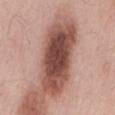workup: no biopsy performed (imaged during a skin exam)
lesion size: about 10 mm
location: the mid back
automated metrics: an outline eccentricity of about 0.95 (0 = round, 1 = elongated) and two-axis asymmetry of about 0.2; border irregularity of about 3.5 on a 0–10 scale, internal color variation of about 6.5 on a 0–10 scale, and a peripheral color-asymmetry measure near 2
patient: male, aged 53 to 57
image: ~15 mm tile from a whole-body skin photo